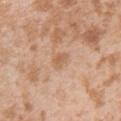This lesion was catalogued during total-body skin photography and was not selected for biopsy. An algorithmic analysis of the crop reported a border-irregularity index near 2.5/10 and radial color variation of about 1.5. A male patient, aged around 25. A 15 mm close-up tile from a total-body photography series done for melanoma screening. The lesion's longest dimension is about 2.5 mm. From the upper back. This is a white-light tile.